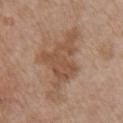Acquisition and patient details:
A 15 mm close-up tile from a total-body photography series done for melanoma screening. A female subject, aged 73 to 77. Located on the chest.